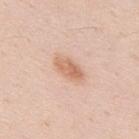This lesion was catalogued during total-body skin photography and was not selected for biopsy.
The subject is a male about 35 years old.
Located on the upper back.
Automated tile analysis of the lesion measured a lesion color around L≈67 a*≈21 b*≈32 in CIELAB, roughly 10 lightness units darker than nearby skin, and a lesion-to-skin contrast of about 7 (normalized; higher = more distinct). And it measured border irregularity of about 2 on a 0–10 scale, a within-lesion color-variation index near 4/10, and a peripheral color-asymmetry measure near 1.5. The analysis additionally found an automated nevus-likeness rating near 90 out of 100 and a lesion-detection confidence of about 100/100.
A 15 mm close-up extracted from a 3D total-body photography capture.
Longest diameter approximately 4 mm.